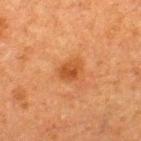Findings:
• notes — total-body-photography surveillance lesion; no biopsy
• location — the upper back
• image — ~15 mm crop, total-body skin-cancer survey
• patient — male, aged 48 to 52